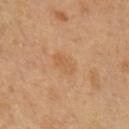Impression: Imaged during a routine full-body skin examination; the lesion was not biopsied and no histopathology is available. Background: The subject is a male aged 48 to 52. The total-body-photography lesion software estimated a lesion area of about 5.5 mm², an eccentricity of roughly 0.8, and a shape-asymmetry score of about 0.25 (0 = symmetric). And it measured a mean CIELAB color near L≈57 a*≈20 b*≈37. And it measured a border-irregularity rating of about 2.5/10, a color-variation rating of about 1.5/10, and peripheral color asymmetry of about 0.5. On the chest. A roughly 15 mm field-of-view crop from a total-body skin photograph. Approximately 3.5 mm at its widest.